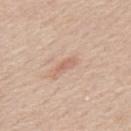Assessment: This lesion was catalogued during total-body skin photography and was not selected for biopsy.